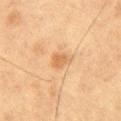Part of a total-body skin-imaging series; this lesion was reviewed on a skin check and was not flagged for biopsy. A lesion tile, about 15 mm wide, cut from a 3D total-body photograph. Located on the right thigh. Captured under cross-polarized illumination. The patient is a male in their mid- to late 50s.